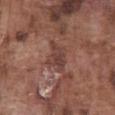Case summary:
* biopsy status — no biopsy performed (imaged during a skin exam)
* image — ~15 mm crop, total-body skin-cancer survey
* subject — male, roughly 75 years of age
* tile lighting — white-light
* site — the abdomen
* size — ~3.5 mm (longest diameter)